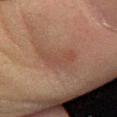Impression:
Imaged during a routine full-body skin examination; the lesion was not biopsied and no histopathology is available.
Background:
Captured under cross-polarized illumination. A roughly 15 mm field-of-view crop from a total-body skin photograph. A male subject aged 58–62. From the leg. Approximately 4.5 mm at its widest.